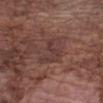Assessment: The lesion was photographed on a routine skin check and not biopsied; there is no pathology result. Context: Automated image analysis of the tile measured an average lesion color of about L≈37 a*≈19 b*≈20 (CIELAB), roughly 6 lightness units darker than nearby skin, and a normalized lesion–skin contrast near 6. And it measured a detector confidence of about 55 out of 100 that the crop contains a lesion. Approximately 4.5 mm at its widest. The lesion is on the left forearm. A lesion tile, about 15 mm wide, cut from a 3D total-body photograph. The subject is a male in their mid-70s.